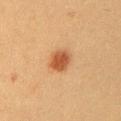Imaged during a routine full-body skin examination; the lesion was not biopsied and no histopathology is available.
The subject is a female roughly 40 years of age.
An algorithmic analysis of the crop reported a lesion color around L≈44 a*≈23 b*≈34 in CIELAB, about 12 CIELAB-L* units darker than the surrounding skin, and a normalized lesion–skin contrast near 9. The analysis additionally found border irregularity of about 1.5 on a 0–10 scale, a within-lesion color-variation index near 2.5/10, and a peripheral color-asymmetry measure near 0.5. It also reported a nevus-likeness score of about 100/100 and a detector confidence of about 100 out of 100 that the crop contains a lesion.
The lesion is located on the left upper arm.
A 15 mm close-up tile from a total-body photography series done for melanoma screening.
The recorded lesion diameter is about 3 mm.
The tile uses cross-polarized illumination.